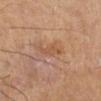Recorded during total-body skin imaging; not selected for excision or biopsy.
From the leg.
A male patient about 60 years old.
A 15 mm close-up extracted from a 3D total-body photography capture.